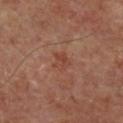No biopsy was performed on this lesion — it was imaged during a full skin examination and was not determined to be concerning.
A male subject aged 63 to 67.
About 2.5 mm across.
The lesion is located on the right lower leg.
The tile uses cross-polarized illumination.
A roughly 15 mm field-of-view crop from a total-body skin photograph.